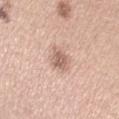biopsy status: catalogued during a skin exam; not biopsied
diameter: ~3 mm (longest diameter)
subject: female, roughly 30 years of age
image source: ~15 mm crop, total-body skin-cancer survey
site: the left upper arm